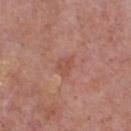Imaged during a routine full-body skin examination; the lesion was not biopsied and no histopathology is available. Automated tile analysis of the lesion measured a shape eccentricity near 0.6. The software also gave a mean CIELAB color near L≈51 a*≈24 b*≈29, a lesion–skin lightness drop of about 6, and a normalized lesion–skin contrast near 5. It also reported a nevus-likeness score of about 0/100 and a detector confidence of about 100 out of 100 that the crop contains a lesion. A male subject, aged 73–77. Cropped from a total-body skin-imaging series; the visible field is about 15 mm. The lesion is located on the chest.